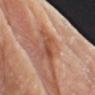Part of a total-body skin-imaging series; this lesion was reviewed on a skin check and was not flagged for biopsy.
Captured under white-light illumination.
The lesion is on the arm.
The subject is a male aged around 80.
A roughly 15 mm field-of-view crop from a total-body skin photograph.